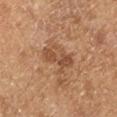Clinical impression:
No biopsy was performed on this lesion — it was imaged during a full skin examination and was not determined to be concerning.
Clinical summary:
The lesion-visualizer software estimated an eccentricity of roughly 0.85 and a shape-asymmetry score of about 0.35 (0 = symmetric). The analysis additionally found a mean CIELAB color near L≈50 a*≈21 b*≈34, a lesion–skin lightness drop of about 9, and a lesion-to-skin contrast of about 6.5 (normalized; higher = more distinct). Approximately 4.5 mm at its widest. A lesion tile, about 15 mm wide, cut from a 3D total-body photograph. From the right lower leg. A male patient, aged approximately 65.